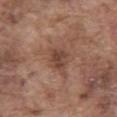Impression:
Captured during whole-body skin photography for melanoma surveillance; the lesion was not biopsied.
Clinical summary:
A 15 mm close-up extracted from a 3D total-body photography capture. A male subject, aged around 75. On the abdomen. Approximately 2.5 mm at its widest. Automated tile analysis of the lesion measured a border-irregularity index near 2.5/10, a within-lesion color-variation index near 2.5/10, and peripheral color asymmetry of about 1. It also reported lesion-presence confidence of about 100/100. The tile uses white-light illumination.